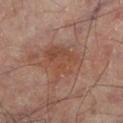lesion diameter = ~5.5 mm (longest diameter) | subject = male, in their mid- to late 60s | tile lighting = cross-polarized illumination | imaging modality = total-body-photography crop, ~15 mm field of view | automated metrics = a border-irregularity rating of about 4/10, a within-lesion color-variation index near 4/10, and peripheral color asymmetry of about 1.5 | body site = the leg.